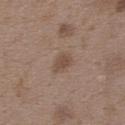<tbp_lesion>
  <biopsy_status>not biopsied; imaged during a skin examination</biopsy_status>
  <patient>
    <sex>female</sex>
    <age_approx>35</age_approx>
  </patient>
  <image>
    <source>total-body photography crop</source>
    <field_of_view_mm>15</field_of_view_mm>
  </image>
  <site>upper back</site>
</tbp_lesion>